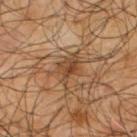Clinical impression: Captured during whole-body skin photography for melanoma surveillance; the lesion was not biopsied. Background: A 15 mm close-up extracted from a 3D total-body photography capture. Approximately 4 mm at its widest. A male patient, aged approximately 65. Captured under cross-polarized illumination. Automated tile analysis of the lesion measured a footprint of about 7.5 mm² and a shape-asymmetry score of about 0.35 (0 = symmetric). The analysis additionally found a color-variation rating of about 5/10 and radial color variation of about 1.5. The analysis additionally found a classifier nevus-likeness of about 5/100 and lesion-presence confidence of about 70/100. The lesion is located on the upper back.